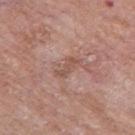Q: Is there a histopathology result?
A: catalogued during a skin exam; not biopsied
Q: What kind of image is this?
A: 15 mm crop, total-body photography
Q: What is the anatomic site?
A: the left thigh
Q: Lesion size?
A: ~3.5 mm (longest diameter)
Q: Who is the patient?
A: male, approximately 80 years of age
Q: How was the tile lit?
A: white-light